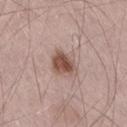biopsy_status: not biopsied; imaged during a skin examination
image:
  source: total-body photography crop
  field_of_view_mm: 15
site: right thigh
patient:
  sex: male
  age_approx: 70
lighting: white-light
automated_metrics:
  eccentricity: 0.5
  shape_asymmetry: 0.25
  nevus_likeness_0_100: 95